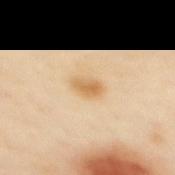Case summary:
- notes: no biopsy performed (imaged during a skin exam)
- automated lesion analysis: an average lesion color of about L≈68 a*≈18 b*≈42 (CIELAB), a lesion–skin lightness drop of about 11, and a lesion-to-skin contrast of about 7.5 (normalized; higher = more distinct); a border-irregularity index near 2/10 and a within-lesion color-variation index near 3.5/10
- anatomic site: the upper back
- lighting: cross-polarized illumination
- patient: female, aged 38 to 42
- imaging modality: ~15 mm tile from a whole-body skin photo